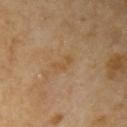Captured during whole-body skin photography for melanoma surveillance; the lesion was not biopsied. A female subject, roughly 70 years of age. Automated image analysis of the tile measured a mean CIELAB color near L≈53 a*≈17 b*≈38, a lesion–skin lightness drop of about 5, and a normalized border contrast of about 5.5. The software also gave lesion-presence confidence of about 100/100. This is a cross-polarized tile. On the right upper arm. This image is a 15 mm lesion crop taken from a total-body photograph. The recorded lesion diameter is about 2.5 mm.